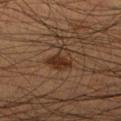| feature | finding |
|---|---|
| workup | no biopsy performed (imaged during a skin exam) |
| body site | the right lower leg |
| illumination | cross-polarized |
| TBP lesion metrics | a footprint of about 7 mm², an outline eccentricity of about 0.4 (0 = round, 1 = elongated), and two-axis asymmetry of about 0.4; a mean CIELAB color near L≈30 a*≈18 b*≈27, a lesion–skin lightness drop of about 8, and a normalized border contrast of about 8; a border-irregularity index near 4/10 and internal color variation of about 6 on a 0–10 scale; a nevus-likeness score of about 95/100 and a detector confidence of about 100 out of 100 that the crop contains a lesion |
| image | total-body-photography crop, ~15 mm field of view |
| patient | male, in their mid-40s |
| lesion diameter | ~3.5 mm (longest diameter) |